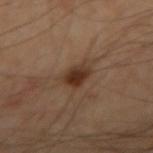Impression: The lesion was tiled from a total-body skin photograph and was not biopsied. Background: Approximately 3 mm at its widest. The lesion is located on the arm. A male subject aged 63 to 67. Captured under cross-polarized illumination. A close-up tile cropped from a whole-body skin photograph, about 15 mm across.